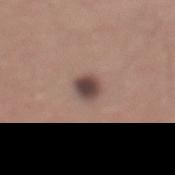No biopsy was performed on this lesion — it was imaged during a full skin examination and was not determined to be concerning.
A 15 mm crop from a total-body photograph taken for skin-cancer surveillance.
A male subject, aged around 45.
Located on the mid back.
Approximately 3 mm at its widest.